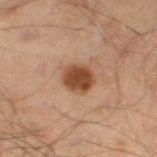The lesion-visualizer software estimated a lesion color around L≈37 a*≈18 b*≈28 in CIELAB, roughly 12 lightness units darker than nearby skin, and a normalized border contrast of about 10.5. And it measured a border-irregularity index near 1.5/10, a within-lesion color-variation index near 2.5/10, and a peripheral color-asymmetry measure near 1. It also reported a detector confidence of about 100 out of 100 that the crop contains a lesion. Located on the left thigh. A male subject, approximately 70 years of age. Captured under cross-polarized illumination. A roughly 15 mm field-of-view crop from a total-body skin photograph.